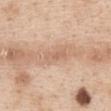* follow-up — catalogued during a skin exam; not biopsied
* lesion size — ≈3.5 mm
* image — ~15 mm crop, total-body skin-cancer survey
* subject — female, approximately 40 years of age
* anatomic site — the chest
* lighting — white-light illumination
* image-analysis metrics — an area of roughly 4.5 mm², a shape eccentricity near 0.9, and two-axis asymmetry of about 0.3